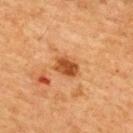follow-up = catalogued during a skin exam; not biopsied | patient = male, approximately 65 years of age | image = total-body-photography crop, ~15 mm field of view | tile lighting = cross-polarized illumination | diameter = ~3 mm (longest diameter) | automated metrics = border irregularity of about 2 on a 0–10 scale, internal color variation of about 3 on a 0–10 scale, and radial color variation of about 1; an automated nevus-likeness rating near 75 out of 100 and a detector confidence of about 100 out of 100 that the crop contains a lesion | site = the upper back.